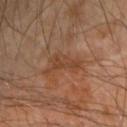The lesion was photographed on a routine skin check and not biopsied; there is no pathology result.
The total-body-photography lesion software estimated a footprint of about 7.5 mm², an outline eccentricity of about 0.9 (0 = round, 1 = elongated), and a shape-asymmetry score of about 0.45 (0 = symmetric). The analysis additionally found a normalized border contrast of about 5.5. It also reported a detector confidence of about 100 out of 100 that the crop contains a lesion.
A male subject roughly 70 years of age.
Approximately 4.5 mm at its widest.
On the right forearm.
A lesion tile, about 15 mm wide, cut from a 3D total-body photograph.
Imaged with cross-polarized lighting.